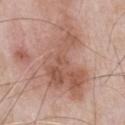Impression:
Part of a total-body skin-imaging series; this lesion was reviewed on a skin check and was not flagged for biopsy.
Context:
A roughly 15 mm field-of-view crop from a total-body skin photograph. Located on the chest. The patient is a male roughly 50 years of age. This is a white-light tile. The recorded lesion diameter is about 11 mm.